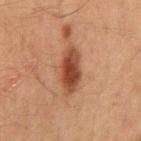Case summary:
• size: ≈5.5 mm
• tile lighting: cross-polarized illumination
• anatomic site: the mid back
• patient: male, roughly 65 years of age
• image: ~15 mm tile from a whole-body skin photo